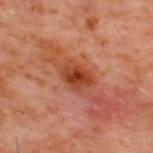Q: Is there a histopathology result?
A: no biopsy performed (imaged during a skin exam)
Q: What lighting was used for the tile?
A: cross-polarized illumination
Q: What kind of image is this?
A: total-body-photography crop, ~15 mm field of view
Q: What is the anatomic site?
A: the back
Q: Patient demographics?
A: male, aged 58–62